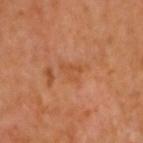Assessment:
Part of a total-body skin-imaging series; this lesion was reviewed on a skin check and was not flagged for biopsy.
Background:
A male patient aged 58 to 62. Cropped from a whole-body photographic skin survey; the tile spans about 15 mm. On the head or neck.